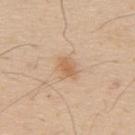Clinical impression: No biopsy was performed on this lesion — it was imaged during a full skin examination and was not determined to be concerning. Image and clinical context: The total-body-photography lesion software estimated a footprint of about 4.5 mm², an outline eccentricity of about 0.75 (0 = round, 1 = elongated), and a symmetry-axis asymmetry near 0.3. The analysis additionally found a mean CIELAB color near L≈62 a*≈19 b*≈35, roughly 9 lightness units darker than nearby skin, and a lesion-to-skin contrast of about 6.5 (normalized; higher = more distinct). The software also gave a classifier nevus-likeness of about 70/100 and a lesion-detection confidence of about 100/100. Approximately 3 mm at its widest. Captured under white-light illumination. A region of skin cropped from a whole-body photographic capture, roughly 15 mm wide. The lesion is on the upper back. A male patient roughly 60 years of age.